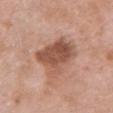A female subject, approximately 70 years of age.
A 15 mm close-up tile from a total-body photography series done for melanoma screening.
The lesion is on the chest.